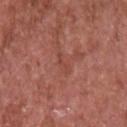follow-up: total-body-photography surveillance lesion; no biopsy.